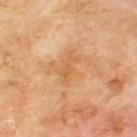A male subject approximately 70 years of age.
An algorithmic analysis of the crop reported lesion-presence confidence of about 100/100.
Located on the upper back.
A roughly 15 mm field-of-view crop from a total-body skin photograph.